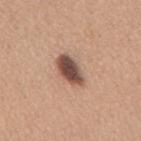Q: Is there a histopathology result?
A: catalogued during a skin exam; not biopsied
Q: What kind of image is this?
A: 15 mm crop, total-body photography
Q: How was the tile lit?
A: white-light illumination
Q: Who is the patient?
A: female, approximately 30 years of age
Q: What is the lesion's diameter?
A: about 4 mm
Q: What is the anatomic site?
A: the back